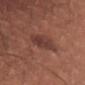Part of a total-body skin-imaging series; this lesion was reviewed on a skin check and was not flagged for biopsy.
About 4 mm across.
The subject is a female in their mid- to late 40s.
A close-up tile cropped from a whole-body skin photograph, about 15 mm across.
From the right thigh.